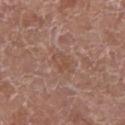<lesion>
<biopsy_status>not biopsied; imaged during a skin examination</biopsy_status>
<site>leg</site>
<patient>
  <sex>female</sex>
  <age_approx>80</age_approx>
</patient>
<image>
  <source>total-body photography crop</source>
  <field_of_view_mm>15</field_of_view_mm>
</image>
</lesion>